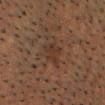Impression: Captured during whole-body skin photography for melanoma surveillance; the lesion was not biopsied. Background: A close-up tile cropped from a whole-body skin photograph, about 15 mm across. From the head or neck. The patient is a male roughly 55 years of age. Approximately 3 mm at its widest.